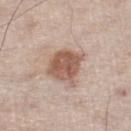follow-up: imaged on a skin check; not biopsied
lighting: white-light illumination
patient: male, roughly 60 years of age
lesion diameter: ≈4 mm
image source: 15 mm crop, total-body photography
location: the leg